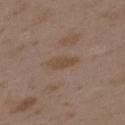Captured during whole-body skin photography for melanoma surveillance; the lesion was not biopsied. Approximately 3.5 mm at its widest. From the upper back. A 15 mm crop from a total-body photograph taken for skin-cancer surveillance. This is a white-light tile. A female subject, aged around 35. Automated image analysis of the tile measured a border-irregularity rating of about 2.5/10, internal color variation of about 1 on a 0–10 scale, and peripheral color asymmetry of about 0.